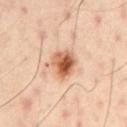Clinical impression: The lesion was photographed on a routine skin check and not biopsied; there is no pathology result. Clinical summary: The lesion is on the left upper arm. Automated image analysis of the tile measured a lesion color around L≈60 a*≈24 b*≈35 in CIELAB, roughly 16 lightness units darker than nearby skin, and a lesion-to-skin contrast of about 10 (normalized; higher = more distinct). And it measured internal color variation of about 9.5 on a 0–10 scale and a peripheral color-asymmetry measure near 3. The analysis additionally found a nevus-likeness score of about 95/100 and a detector confidence of about 100 out of 100 that the crop contains a lesion. A male patient, aged approximately 50. A lesion tile, about 15 mm wide, cut from a 3D total-body photograph. Measured at roughly 3.5 mm in maximum diameter. Captured under cross-polarized illumination.